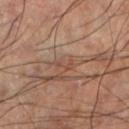Impression:
Recorded during total-body skin imaging; not selected for excision or biopsy.
Context:
Automated image analysis of the tile measured two-axis asymmetry of about 0.5. The software also gave a lesion color around L≈46 a*≈20 b*≈27 in CIELAB and roughly 6 lightness units darker than nearby skin. The analysis additionally found a border-irregularity index near 6.5/10, a color-variation rating of about 0/10, and a peripheral color-asymmetry measure near 0. Located on the left lower leg. Approximately 2.5 mm at its widest. A close-up tile cropped from a whole-body skin photograph, about 15 mm across. A male subject, aged 58 to 62. Captured under cross-polarized illumination.